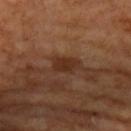The lesion was tiled from a total-body skin photograph and was not biopsied. About 3.5 mm across. Located on the left upper arm. Captured under cross-polarized illumination. The patient is a male roughly 55 years of age. An algorithmic analysis of the crop reported an outline eccentricity of about 0.8 (0 = round, 1 = elongated) and two-axis asymmetry of about 0.25. The software also gave border irregularity of about 2.5 on a 0–10 scale and a peripheral color-asymmetry measure near 1. A close-up tile cropped from a whole-body skin photograph, about 15 mm across.